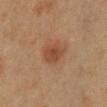About 3.5 mm across. The lesion is on the mid back. A male subject roughly 60 years of age. Cropped from a total-body skin-imaging series; the visible field is about 15 mm.